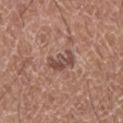Findings:
– workup · catalogued during a skin exam; not biopsied
– size · about 3.5 mm
– body site · the left lower leg
– image source · ~15 mm crop, total-body skin-cancer survey
– tile lighting · white-light
– subject · female, aged around 50
– TBP lesion metrics · a lesion color around L≈48 a*≈20 b*≈24 in CIELAB, about 10 CIELAB-L* units darker than the surrounding skin, and a normalized border contrast of about 7.5; border irregularity of about 5 on a 0–10 scale and a peripheral color-asymmetry measure near 1; a nevus-likeness score of about 0/100 and a lesion-detection confidence of about 100/100